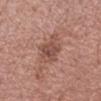follow-up: imaged on a skin check; not biopsied | location: the arm | image-analysis metrics: an average lesion color of about L≈50 a*≈22 b*≈26 (CIELAB); border irregularity of about 4.5 on a 0–10 scale; an automated nevus-likeness rating near 0 out of 100 and lesion-presence confidence of about 100/100 | imaging modality: 15 mm crop, total-body photography | lesion size: ≈4 mm | subject: female, aged 53–57.